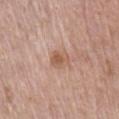Assessment:
Part of a total-body skin-imaging series; this lesion was reviewed on a skin check and was not flagged for biopsy.
Context:
A close-up tile cropped from a whole-body skin photograph, about 15 mm across. Located on the chest. A male patient, aged 68–72.